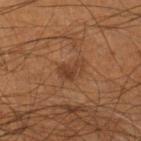biopsy status — no biopsy performed (imaged during a skin exam) | automated metrics — border irregularity of about 3.5 on a 0–10 scale, a within-lesion color-variation index near 2/10, and peripheral color asymmetry of about 0.5; a nevus-likeness score of about 10/100 and lesion-presence confidence of about 100/100 | location — the right lower leg | subject — male, aged 53–57 | image — 15 mm crop, total-body photography | lesion diameter — ~3 mm (longest diameter).